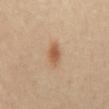<tbp_lesion>
  <biopsy_status>not biopsied; imaged during a skin examination</biopsy_status>
  <lesion_size>
    <long_diameter_mm_approx>3.0</long_diameter_mm_approx>
  </lesion_size>
  <patient>
    <sex>male</sex>
    <age_approx>60</age_approx>
  </patient>
  <automated_metrics>
    <area_mm2_approx>4.5</area_mm2_approx>
    <eccentricity>0.8</eccentricity>
    <shape_asymmetry>0.25</shape_asymmetry>
    <border_irregularity_0_10>2.5</border_irregularity_0_10>
    <color_variation_0_10>3.5</color_variation_0_10>
    <peripheral_color_asymmetry>1.0</peripheral_color_asymmetry>
  </automated_metrics>
  <image>
    <source>total-body photography crop</source>
    <field_of_view_mm>15</field_of_view_mm>
  </image>
  <lighting>cross-polarized</lighting>
  <site>abdomen</site>
</tbp_lesion>